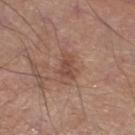Assessment: Captured during whole-body skin photography for melanoma surveillance; the lesion was not biopsied. Clinical summary: This image is a 15 mm lesion crop taken from a total-body photograph. This is a white-light tile. A male subject, about 70 years old. From the left lower leg. About 3 mm across.